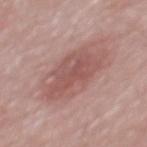Part of a total-body skin-imaging series; this lesion was reviewed on a skin check and was not flagged for biopsy. Captured under white-light illumination. The patient is a male aged 48–52. The lesion's longest dimension is about 6.5 mm. This image is a 15 mm lesion crop taken from a total-body photograph. Automated image analysis of the tile measured a lesion–skin lightness drop of about 9 and a lesion-to-skin contrast of about 6 (normalized; higher = more distinct). And it measured border irregularity of about 4 on a 0–10 scale, a within-lesion color-variation index near 3/10, and radial color variation of about 1. The lesion is on the back.